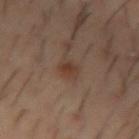Imaged during a routine full-body skin examination; the lesion was not biopsied and no histopathology is available.
The tile uses cross-polarized illumination.
About 3.5 mm across.
From the left forearm.
A male subject about 55 years old.
A 15 mm close-up tile from a total-body photography series done for melanoma screening.